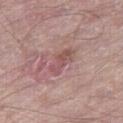Assessment: No biopsy was performed on this lesion — it was imaged during a full skin examination and was not determined to be concerning. Context: On the right thigh. Cropped from a total-body skin-imaging series; the visible field is about 15 mm. Imaged with white-light lighting. A male subject, aged 63 to 67.